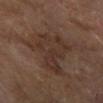biopsy status = imaged on a skin check; not biopsied
tile lighting = cross-polarized
subject = female, aged approximately 60
automated metrics = a border-irregularity rating of about 5.5/10, a color-variation rating of about 3/10, and radial color variation of about 1; a classifier nevus-likeness of about 0/100 and a detector confidence of about 100 out of 100 that the crop contains a lesion
diameter = about 7 mm
anatomic site = the right forearm
imaging modality = 15 mm crop, total-body photography